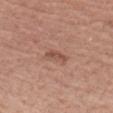subject = male, in their mid- to late 60s; location = the left forearm; diameter = about 3 mm; imaging modality = ~15 mm crop, total-body skin-cancer survey; tile lighting = white-light illumination.